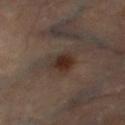| field | value |
|---|---|
| biopsy status | total-body-photography surveillance lesion; no biopsy |
| acquisition | ~15 mm crop, total-body skin-cancer survey |
| body site | the right lower leg |
| patient | male, in their 70s |